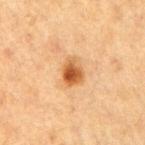Part of a total-body skin-imaging series; this lesion was reviewed on a skin check and was not flagged for biopsy.
Measured at roughly 3 mm in maximum diameter.
A 15 mm crop from a total-body photograph taken for skin-cancer surveillance.
Automated image analysis of the tile measured a lesion-detection confidence of about 100/100.
From the right upper arm.
The subject is a female aged 38 to 42.
The tile uses cross-polarized illumination.